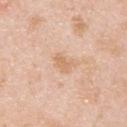Recorded during total-body skin imaging; not selected for excision or biopsy. On the upper back. A male patient in their mid-20s. This image is a 15 mm lesion crop taken from a total-body photograph.